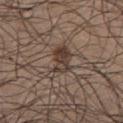notes — imaged on a skin check; not biopsied
location — the chest
image source — total-body-photography crop, ~15 mm field of view
subject — male, aged 23–27